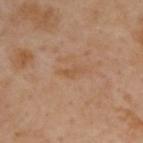* workup — no biopsy performed (imaged during a skin exam)
* anatomic site — the upper back
* patient — male, aged 53–57
* acquisition — ~15 mm crop, total-body skin-cancer survey
* automated metrics — a lesion–skin lightness drop of about 6 and a normalized border contrast of about 5.5; a border-irregularity index near 5/10, a color-variation rating of about 0/10, and peripheral color asymmetry of about 0; an automated nevus-likeness rating near 0 out of 100 and a lesion-detection confidence of about 100/100
* illumination — cross-polarized